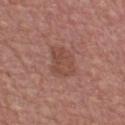Clinical impression: Imaged during a routine full-body skin examination; the lesion was not biopsied and no histopathology is available. Acquisition and patient details: Located on the chest. A 15 mm crop from a total-body photograph taken for skin-cancer surveillance. The subject is a male aged around 70. The total-body-photography lesion software estimated a lesion-detection confidence of about 100/100. The tile uses white-light illumination.